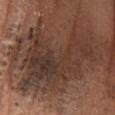  automated_metrics:
    cielab_L: 34
    cielab_a: 16
    cielab_b: 23
    vs_skin_darker_L: 8.0
    vs_skin_contrast_norm: 7.5
    border_irregularity_0_10: 9.0
    peripheral_color_asymmetry: 2.0
  site: left lower leg
  image:
    source: total-body photography crop
    field_of_view_mm: 15
  patient:
    sex: male
    age_approx: 65
  lighting: cross-polarized